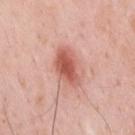Impression: Recorded during total-body skin imaging; not selected for excision or biopsy. Clinical summary: The lesion-visualizer software estimated a lesion color around L≈58 a*≈29 b*≈29 in CIELAB, about 13 CIELAB-L* units darker than the surrounding skin, and a normalized lesion–skin contrast near 8.5. It also reported an automated nevus-likeness rating near 95 out of 100 and a lesion-detection confidence of about 100/100. A male patient aged around 35. This image is a 15 mm lesion crop taken from a total-body photograph. The lesion is on the upper back. Approximately 4.5 mm at its widest. Imaged with white-light lighting.